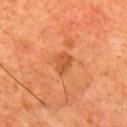Captured under cross-polarized illumination. From the back. About 2.5 mm across. A region of skin cropped from a whole-body photographic capture, roughly 15 mm wide. The subject is a male aged 58 to 62.